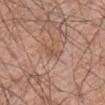notes=imaged on a skin check; not biopsied
size=~3 mm (longest diameter)
body site=the chest
image-analysis metrics=a lesion color around L≈53 a*≈20 b*≈28 in CIELAB and about 8 CIELAB-L* units darker than the surrounding skin; border irregularity of about 4 on a 0–10 scale, internal color variation of about 1.5 on a 0–10 scale, and radial color variation of about 0.5
illumination=white-light
subject=male, in their 70s
acquisition=total-body-photography crop, ~15 mm field of view